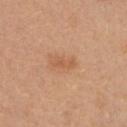Imaged during a routine full-body skin examination; the lesion was not biopsied and no histopathology is available. The lesion's longest dimension is about 3 mm. Imaged with white-light lighting. A female subject in their mid-20s. A close-up tile cropped from a whole-body skin photograph, about 15 mm across. From the chest.